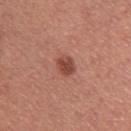The lesion was tiled from a total-body skin photograph and was not biopsied. The subject is a male in their 40s. This is a white-light tile. The lesion is located on the left upper arm. The recorded lesion diameter is about 2.5 mm. A 15 mm close-up extracted from a 3D total-body photography capture.